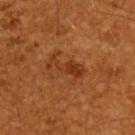patient: male, aged around 60; image source: ~15 mm crop, total-body skin-cancer survey; anatomic site: the upper back; diameter: ≈4 mm; tile lighting: cross-polarized illumination.